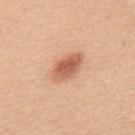  biopsy_status: not biopsied; imaged during a skin examination
  lesion_size:
    long_diameter_mm_approx: 4.5
  patient:
    sex: male
    age_approx: 50
  image:
    source: total-body photography crop
    field_of_view_mm: 15
  automated_metrics:
    area_mm2_approx: 9.0
    eccentricity: 0.85
    shape_asymmetry: 0.15
    cielab_L: 61
    cielab_a: 25
    cielab_b: 34
    vs_skin_darker_L: 13.0
    vs_skin_contrast_norm: 8.0
    nevus_likeness_0_100: 100
    lesion_detection_confidence_0_100: 100
  site: upper back
  lighting: white-light